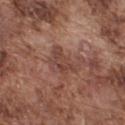Imaged during a routine full-body skin examination; the lesion was not biopsied and no histopathology is available.
An algorithmic analysis of the crop reported an eccentricity of roughly 0.7 and two-axis asymmetry of about 0.45. The software also gave a border-irregularity index near 5/10, internal color variation of about 3 on a 0–10 scale, and peripheral color asymmetry of about 1. It also reported a nevus-likeness score of about 0/100.
A male subject approximately 75 years of age.
The lesion is located on the upper back.
A roughly 15 mm field-of-view crop from a total-body skin photograph.
The lesion's longest dimension is about 4 mm.
The tile uses white-light illumination.